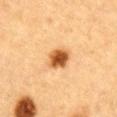<lesion>
<biopsy_status>not biopsied; imaged during a skin examination</biopsy_status>
<automated_metrics>
  <cielab_L>51</cielab_L>
  <cielab_a>23</cielab_a>
  <cielab_b>40</cielab_b>
  <vs_skin_contrast_norm>11.0</vs_skin_contrast_norm>
  <nevus_likeness_0_100>100</nevus_likeness_0_100>
  <lesion_detection_confidence_0_100>100</lesion_detection_confidence_0_100>
</automated_metrics>
<lighting>cross-polarized</lighting>
<site>abdomen</site>
<patient>
  <sex>male</sex>
  <age_approx>85+</age_approx>
</patient>
<lesion_size>
  <long_diameter_mm_approx>3.0</long_diameter_mm_approx>
</lesion_size>
<image>
  <source>total-body photography crop</source>
  <field_of_view_mm>15</field_of_view_mm>
</image>
</lesion>